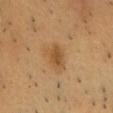Captured during whole-body skin photography for melanoma surveillance; the lesion was not biopsied. A male patient, roughly 60 years of age. Captured under cross-polarized illumination. About 3 mm across. The total-body-photography lesion software estimated an outline eccentricity of about 0.75 (0 = round, 1 = elongated) and a shape-asymmetry score of about 0.3 (0 = symmetric). The software also gave a border-irregularity rating of about 2.5/10, a within-lesion color-variation index near 2/10, and peripheral color asymmetry of about 1. The lesion is located on the chest. A lesion tile, about 15 mm wide, cut from a 3D total-body photograph.